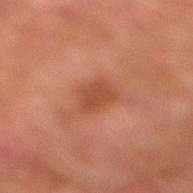<lesion>
<biopsy_status>not biopsied; imaged during a skin examination</biopsy_status>
<image>
  <source>total-body photography crop</source>
  <field_of_view_mm>15</field_of_view_mm>
</image>
<site>leg</site>
<patient>
  <sex>male</sex>
  <age_approx>75</age_approx>
</patient>
</lesion>